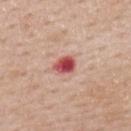The lesion was tiled from a total-body skin photograph and was not biopsied. A male subject, aged 58 to 62. On the upper back. This image is a 15 mm lesion crop taken from a total-body photograph.